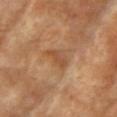Impression: This lesion was catalogued during total-body skin photography and was not selected for biopsy. Background: A lesion tile, about 15 mm wide, cut from a 3D total-body photograph. Captured under cross-polarized illumination. About 3.5 mm across. The lesion-visualizer software estimated a within-lesion color-variation index near 2/10. The patient is a female about 75 years old. On the left upper arm.